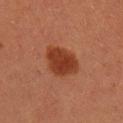– anatomic site · the leg
– patient · female, aged 38 to 42
– image source · 15 mm crop, total-body photography